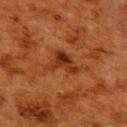Q: Is there a histopathology result?
A: catalogued during a skin exam; not biopsied
Q: What are the patient's age and sex?
A: female, about 50 years old
Q: What kind of image is this?
A: ~15 mm tile from a whole-body skin photo
Q: Lesion location?
A: the upper back